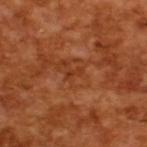The lesion was tiled from a total-body skin photograph and was not biopsied. A roughly 15 mm field-of-view crop from a total-body skin photograph. Captured under cross-polarized illumination. The recorded lesion diameter is about 2 mm. A male subject, aged approximately 65.